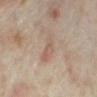The lesion was tiled from a total-body skin photograph and was not biopsied. A female patient aged around 35. This is a cross-polarized tile. A roughly 15 mm field-of-view crop from a total-body skin photograph. The lesion is on the right forearm. Longest diameter approximately 4 mm.